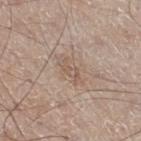{
  "lighting": "white-light",
  "image": {
    "source": "total-body photography crop",
    "field_of_view_mm": 15
  },
  "site": "leg",
  "lesion_size": {
    "long_diameter_mm_approx": 2.5
  },
  "automated_metrics": {
    "vs_skin_contrast_norm": 5.0,
    "nevus_likeness_0_100": 0,
    "lesion_detection_confidence_0_100": 100
  },
  "patient": {
    "sex": "male",
    "age_approx": 60
  }
}